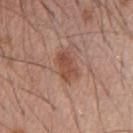Notes:
• notes — catalogued during a skin exam; not biopsied
• body site — the chest
• patient — male, aged 63 to 67
• image source — total-body-photography crop, ~15 mm field of view